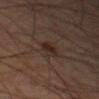* follow-up — no biopsy performed (imaged during a skin exam)
* diameter — ≈3 mm
* acquisition — ~15 mm tile from a whole-body skin photo
* body site — the right forearm
* illumination — cross-polarized
* subject — male, in their mid-40s
* automated metrics — a mean CIELAB color near L≈24 a*≈13 b*≈20, about 6 CIELAB-L* units darker than the surrounding skin, and a normalized border contrast of about 7.5; an automated nevus-likeness rating near 55 out of 100 and a lesion-detection confidence of about 100/100